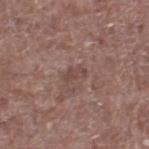– follow-up — imaged on a skin check; not biopsied
– tile lighting — white-light
– lesion size — about 2.5 mm
– automated lesion analysis — a footprint of about 2.5 mm², an eccentricity of roughly 0.85, and a shape-asymmetry score of about 0.45 (0 = symmetric); a mean CIELAB color near L≈44 a*≈19 b*≈21, a lesion–skin lightness drop of about 7, and a normalized border contrast of about 6
– patient — male, in their 70s
– image source — ~15 mm tile from a whole-body skin photo
– location — the right lower leg